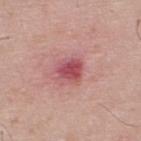Captured under white-light illumination.
The lesion is located on the upper back.
The patient is a male aged 38 to 42.
Automated image analysis of the tile measured a lesion area of about 6 mm², an outline eccentricity of about 0.45 (0 = round, 1 = elongated), and two-axis asymmetry of about 0.25. It also reported a lesion color around L≈51 a*≈33 b*≈20 in CIELAB, a lesion–skin lightness drop of about 13, and a lesion-to-skin contrast of about 8.5 (normalized; higher = more distinct).
A 15 mm crop from a total-body photograph taken for skin-cancer surveillance.